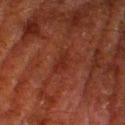workup=imaged on a skin check; not biopsied | site=the right thigh | patient=male, aged around 80 | imaging modality=15 mm crop, total-body photography.